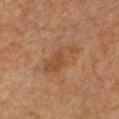The lesion was tiled from a total-body skin photograph and was not biopsied.
Imaged with cross-polarized lighting.
The lesion-visualizer software estimated an area of roughly 8.5 mm² and a shape eccentricity near 0.9. It also reported a lesion color around L≈40 a*≈19 b*≈30 in CIELAB, about 6 CIELAB-L* units darker than the surrounding skin, and a normalized border contrast of about 6.
The lesion's longest dimension is about 5 mm.
The lesion is located on the chest.
Cropped from a total-body skin-imaging series; the visible field is about 15 mm.
A female subject, approximately 50 years of age.